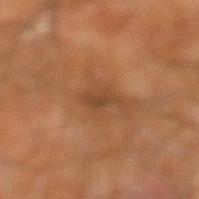  biopsy_status: not biopsied; imaged during a skin examination
  image:
    source: total-body photography crop
    field_of_view_mm: 15
  lesion_size:
    long_diameter_mm_approx: 3.5
  lighting: cross-polarized
  site: right leg
  patient:
    sex: male
    age_approx: 60
  automated_metrics:
    shape_asymmetry: 0.5
    border_irregularity_0_10: 5.0
    color_variation_0_10: 1.0
    peripheral_color_asymmetry: 0.5
    nevus_likeness_0_100: 0
    lesion_detection_confidence_0_100: 90